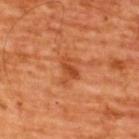{"biopsy_status": "not biopsied; imaged during a skin examination", "site": "upper back", "image": {"source": "total-body photography crop", "field_of_view_mm": 15}, "patient": {"sex": "male", "age_approx": 65}, "automated_metrics": {"area_mm2_approx": 5.5, "eccentricity": 0.7, "shape_asymmetry": 0.45, "color_variation_0_10": 3.5, "peripheral_color_asymmetry": 1.0, "lesion_detection_confidence_0_100": 100}, "lighting": "cross-polarized"}